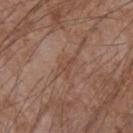{
  "biopsy_status": "not biopsied; imaged during a skin examination",
  "site": "left forearm",
  "lighting": "white-light",
  "automated_metrics": {
    "cielab_L": 46,
    "cielab_a": 19,
    "cielab_b": 27,
    "vs_skin_darker_L": 6.0
  },
  "image": {
    "source": "total-body photography crop",
    "field_of_view_mm": 15
  },
  "patient": {
    "sex": "male",
    "age_approx": 75
  }
}